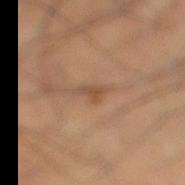Imaged during a routine full-body skin examination; the lesion was not biopsied and no histopathology is available. From the right lower leg. Automated tile analysis of the lesion measured a lesion area of about 2.5 mm², a shape eccentricity near 0.9, and a shape-asymmetry score of about 0.55 (0 = symmetric). And it measured a lesion color around L≈48 a*≈18 b*≈31 in CIELAB and about 8 CIELAB-L* units darker than the surrounding skin. It also reported a border-irregularity rating of about 6/10 and radial color variation of about 0. This is a cross-polarized tile. A male patient approximately 40 years of age. The lesion's longest dimension is about 3 mm. A roughly 15 mm field-of-view crop from a total-body skin photograph.